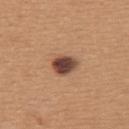The lesion was photographed on a routine skin check and not biopsied; there is no pathology result. A close-up tile cropped from a whole-body skin photograph, about 15 mm across. A female subject, aged 28 to 32. From the upper back. Automated image analysis of the tile measured an average lesion color of about L≈46 a*≈21 b*≈27 (CIELAB) and a normalized lesion–skin contrast near 12.5. It also reported a classifier nevus-likeness of about 85/100 and a detector confidence of about 100 out of 100 that the crop contains a lesion.